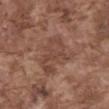biopsy status: no biopsy performed (imaged during a skin exam) | anatomic site: the abdomen | image: ~15 mm tile from a whole-body skin photo | patient: male, approximately 75 years of age | size: about 5 mm | tile lighting: white-light | image-analysis metrics: a footprint of about 18 mm², an outline eccentricity of about 0.4 (0 = round, 1 = elongated), and a symmetry-axis asymmetry near 0.4; a peripheral color-asymmetry measure near 1.5; a detector confidence of about 85 out of 100 that the crop contains a lesion.